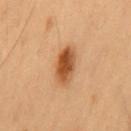Recorded during total-body skin imaging; not selected for excision or biopsy. From the mid back. A male subject aged 53 to 57. The total-body-photography lesion software estimated a mean CIELAB color near L≈42 a*≈20 b*≈33, about 12 CIELAB-L* units darker than the surrounding skin, and a normalized border contrast of about 10. The software also gave a color-variation rating of about 5.5/10 and peripheral color asymmetry of about 1.5. And it measured a nevus-likeness score of about 100/100 and a detector confidence of about 100 out of 100 that the crop contains a lesion. About 4 mm across. This image is a 15 mm lesion crop taken from a total-body photograph.